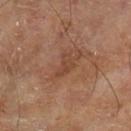Q: Was this lesion biopsied?
A: catalogued during a skin exam; not biopsied
Q: How was the tile lit?
A: cross-polarized illumination
Q: Lesion size?
A: ~3 mm (longest diameter)
Q: Lesion location?
A: the left lower leg
Q: Who is the patient?
A: male, aged approximately 70
Q: What kind of image is this?
A: ~15 mm crop, total-body skin-cancer survey
Q: What did automated image analysis measure?
A: a lesion area of about 4 mm² and an outline eccentricity of about 0.85 (0 = round, 1 = elongated); a border-irregularity rating of about 5.5/10 and a within-lesion color-variation index near 1.5/10; a classifier nevus-likeness of about 0/100